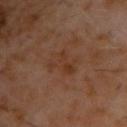Notes:
- site — the chest
- image source — ~15 mm tile from a whole-body skin photo
- patient — male, about 60 years old
- automated lesion analysis — an outline eccentricity of about 0.8 (0 = round, 1 = elongated) and a symmetry-axis asymmetry near 0.3; internal color variation of about 4 on a 0–10 scale